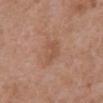<case>
<image>
  <source>total-body photography crop</source>
  <field_of_view_mm>15</field_of_view_mm>
</image>
<site>abdomen</site>
<patient>
  <sex>male</sex>
  <age_approx>70</age_approx>
</patient>
<lighting>white-light</lighting>
</case>